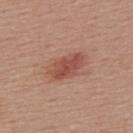A male patient, roughly 55 years of age.
Located on the upper back.
The tile uses white-light illumination.
About 4.5 mm across.
Cropped from a whole-body photographic skin survey; the tile spans about 15 mm.